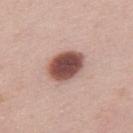Clinical summary: A female patient roughly 50 years of age. Cropped from a whole-body photographic skin survey; the tile spans about 15 mm. The total-body-photography lesion software estimated an average lesion color of about L≈49 a*≈22 b*≈23 (CIELAB) and a normalized lesion–skin contrast near 13. The software also gave border irregularity of about 1 on a 0–10 scale, a within-lesion color-variation index near 5/10, and a peripheral color-asymmetry measure near 1.5. The software also gave a nevus-likeness score of about 90/100 and lesion-presence confidence of about 100/100. On the upper back. Longest diameter approximately 4.5 mm. Diagnosis: On biopsy, histopathology showed a dysplastic (Clark) nevus.